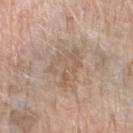<tbp_lesion>
  <biopsy_status>not biopsied; imaged during a skin examination</biopsy_status>
  <site>right forearm</site>
  <patient>
    <sex>female</sex>
    <age_approx>75</age_approx>
  </patient>
  <image>
    <source>total-body photography crop</source>
    <field_of_view_mm>15</field_of_view_mm>
  </image>
  <lighting>white-light</lighting>
  <lesion_size>
    <long_diameter_mm_approx>5.0</long_diameter_mm_approx>
  </lesion_size>
</tbp_lesion>